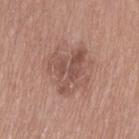Notes:
* notes — no biopsy performed (imaged during a skin exam)
* location — the leg
* TBP lesion metrics — a mean CIELAB color near L≈52 a*≈20 b*≈25, a lesion–skin lightness drop of about 9, and a lesion-to-skin contrast of about 6 (normalized; higher = more distinct); a border-irregularity rating of about 5/10, internal color variation of about 4.5 on a 0–10 scale, and radial color variation of about 2; lesion-presence confidence of about 100/100
* image — total-body-photography crop, ~15 mm field of view
* patient — female, aged 53–57
* lighting — white-light